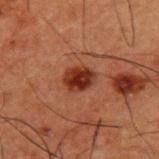Impression:
Captured during whole-body skin photography for melanoma surveillance; the lesion was not biopsied.
Context:
A close-up tile cropped from a whole-body skin photograph, about 15 mm across. From the upper back. A male patient, aged around 50.